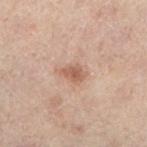Impression:
This lesion was catalogued during total-body skin photography and was not selected for biopsy.
Image and clinical context:
The lesion is located on the left lower leg. A female subject in their mid- to late 50s. This is a cross-polarized tile. A lesion tile, about 15 mm wide, cut from a 3D total-body photograph. The lesion's longest dimension is about 3.5 mm.